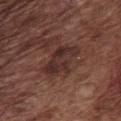No biopsy was performed on this lesion — it was imaged during a full skin examination and was not determined to be concerning. On the chest. Approximately 5 mm at its widest. A male subject, about 75 years old. This image is a 15 mm lesion crop taken from a total-body photograph.